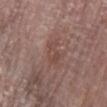Assessment:
The lesion was tiled from a total-body skin photograph and was not biopsied.